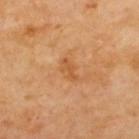Captured during whole-body skin photography for melanoma surveillance; the lesion was not biopsied. A male subject about 70 years old. About 2.5 mm across. A 15 mm close-up extracted from a 3D total-body photography capture. Located on the upper back.